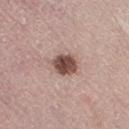{
  "biopsy_status": "not biopsied; imaged during a skin examination",
  "site": "right thigh",
  "lighting": "white-light",
  "image": {
    "source": "total-body photography crop",
    "field_of_view_mm": 15
  },
  "patient": {
    "sex": "female",
    "age_approx": 50
  }
}